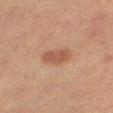follow-up: catalogued during a skin exam; not biopsied | lesion size: ≈4 mm | subject: female, roughly 55 years of age | image: ~15 mm crop, total-body skin-cancer survey | automated metrics: a lesion area of about 7 mm², an outline eccentricity of about 0.85 (0 = round, 1 = elongated), and a symmetry-axis asymmetry near 0.3; about 8 CIELAB-L* units darker than the surrounding skin | site: the right thigh | illumination: cross-polarized.